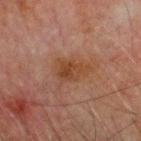workup: no biopsy performed (imaged during a skin exam) | acquisition: 15 mm crop, total-body photography | body site: the head or neck | automated lesion analysis: an area of roughly 7.5 mm², an outline eccentricity of about 0.7 (0 = round, 1 = elongated), and two-axis asymmetry of about 0.3; an average lesion color of about L≈34 a*≈18 b*≈27 (CIELAB) and a lesion–skin lightness drop of about 6; a lesion-detection confidence of about 100/100 | patient: male, roughly 65 years of age | illumination: cross-polarized illumination | lesion size: ≈4 mm.